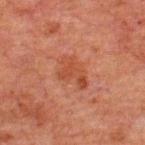notes: imaged on a skin check; not biopsied
image: 15 mm crop, total-body photography
location: the back
size: ≈4 mm
illumination: cross-polarized illumination
patient: male, aged around 60